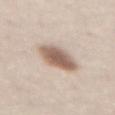Q: Was this lesion biopsied?
A: no biopsy performed (imaged during a skin exam)
Q: Illumination type?
A: white-light illumination
Q: How large is the lesion?
A: ≈4.5 mm
Q: What kind of image is this?
A: ~15 mm tile from a whole-body skin photo
Q: What is the anatomic site?
A: the abdomen
Q: Patient demographics?
A: female, aged 58–62